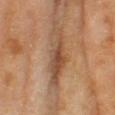Recorded during total-body skin imaging; not selected for excision or biopsy.
A female subject, about 60 years old.
A close-up tile cropped from a whole-body skin photograph, about 15 mm across.
The lesion's longest dimension is about 7 mm.
Located on the left thigh.